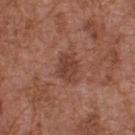Case summary:
– workup — total-body-photography surveillance lesion; no biopsy
– patient — male, aged 73–77
– acquisition — ~15 mm tile from a whole-body skin photo
– location — the upper back
– diameter — about 3 mm